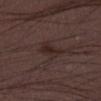{
  "biopsy_status": "not biopsied; imaged during a skin examination",
  "lighting": "white-light",
  "image": {
    "source": "total-body photography crop",
    "field_of_view_mm": 15
  },
  "patient": {
    "sex": "male",
    "age_approx": 50
  },
  "site": "left lower leg"
}